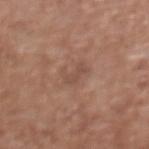| key | value |
|---|---|
| workup | no biopsy performed (imaged during a skin exam) |
| illumination | white-light illumination |
| subject | female, in their mid- to late 70s |
| acquisition | total-body-photography crop, ~15 mm field of view |
| lesion size | ~3 mm (longest diameter) |
| site | the upper back |